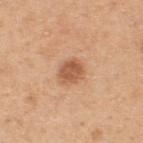{
  "biopsy_status": "not biopsied; imaged during a skin examination",
  "site": "upper back",
  "patient": {
    "sex": "male",
    "age_approx": 50
  },
  "image": {
    "source": "total-body photography crop",
    "field_of_view_mm": 15
  }
}